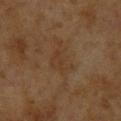Q: Is there a histopathology result?
A: total-body-photography surveillance lesion; no biopsy